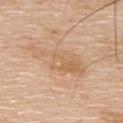Clinical impression:
This lesion was catalogued during total-body skin photography and was not selected for biopsy.
Background:
A male patient, in their mid- to late 70s. Automated tile analysis of the lesion measured a lesion area of about 16 mm², an outline eccentricity of about 0.9 (0 = round, 1 = elongated), and a shape-asymmetry score of about 0.35 (0 = symmetric). The analysis additionally found a lesion color around L≈64 a*≈18 b*≈35 in CIELAB and roughly 7 lightness units darker than nearby skin. And it measured border irregularity of about 5.5 on a 0–10 scale, internal color variation of about 4 on a 0–10 scale, and a peripheral color-asymmetry measure near 1.5. On the upper back. Captured under white-light illumination. A lesion tile, about 15 mm wide, cut from a 3D total-body photograph. Longest diameter approximately 7 mm.